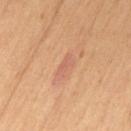  biopsy_status: not biopsied; imaged during a skin examination
  site: lower back
  image:
    source: total-body photography crop
    field_of_view_mm: 15
  patient:
    sex: female
    age_approx: 65
  lesion_size:
    long_diameter_mm_approx: 3.0
  lighting: cross-polarized